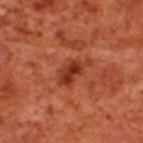No biopsy was performed on this lesion — it was imaged during a full skin examination and was not determined to be concerning. The recorded lesion diameter is about 4.5 mm. The subject is a male aged 68–72. Automated tile analysis of the lesion measured a nevus-likeness score of about 10/100. Imaged with cross-polarized lighting. The lesion is located on the upper back. This image is a 15 mm lesion crop taken from a total-body photograph.